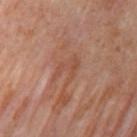This lesion was catalogued during total-body skin photography and was not selected for biopsy. This is a cross-polarized tile. A female patient in their 50s. Automated tile analysis of the lesion measured an automated nevus-likeness rating near 0 out of 100 and lesion-presence confidence of about 55/100. Located on the right upper arm. A roughly 15 mm field-of-view crop from a total-body skin photograph.